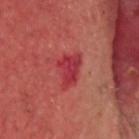notes = catalogued during a skin exam; not biopsied
location = the head or neck
automated lesion analysis = a mean CIELAB color near L≈38 a*≈40 b*≈24 and about 9 CIELAB-L* units darker than the surrounding skin; border irregularity of about 4 on a 0–10 scale, internal color variation of about 2.5 on a 0–10 scale, and a peripheral color-asymmetry measure near 1; a nevus-likeness score of about 0/100 and a detector confidence of about 100 out of 100 that the crop contains a lesion
subject = male, about 65 years old
lesion diameter = about 4 mm
image = ~15 mm crop, total-body skin-cancer survey
tile lighting = cross-polarized